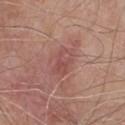A male patient, aged around 80. A roughly 15 mm field-of-view crop from a total-body skin photograph. Measured at roughly 3.5 mm in maximum diameter. From the chest.